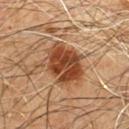biopsy_status: not biopsied; imaged during a skin examination